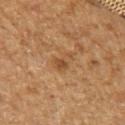Image and clinical context:
The total-body-photography lesion software estimated an area of roughly 4 mm² and an outline eccentricity of about 0.75 (0 = round, 1 = elongated). The analysis additionally found border irregularity of about 5 on a 0–10 scale, a within-lesion color-variation index near 1.5/10, and a peripheral color-asymmetry measure near 0.5. And it measured an automated nevus-likeness rating near 0 out of 100 and a lesion-detection confidence of about 100/100. Measured at roughly 2.5 mm in maximum diameter. A male subject, approximately 65 years of age. Cropped from a total-body skin-imaging series; the visible field is about 15 mm. Located on the arm. Imaged with cross-polarized lighting.